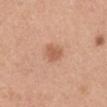biopsy_status: not biopsied; imaged during a skin examination
image:
  source: total-body photography crop
  field_of_view_mm: 15
patient:
  sex: male
  age_approx: 30
site: right upper arm